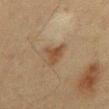Impression: The lesion was photographed on a routine skin check and not biopsied; there is no pathology result. Image and clinical context: A roughly 15 mm field-of-view crop from a total-body skin photograph. Imaged with cross-polarized lighting. The subject is a male approximately 60 years of age. The lesion is on the chest. The lesion's longest dimension is about 3 mm.